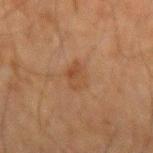This lesion was catalogued during total-body skin photography and was not selected for biopsy. A lesion tile, about 15 mm wide, cut from a 3D total-body photograph. The lesion is located on the left forearm. The patient is a male in their mid- to late 60s.